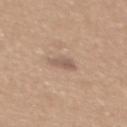Assessment: This lesion was catalogued during total-body skin photography and was not selected for biopsy. Background: On the mid back. A female patient in their 40s. Automated tile analysis of the lesion measured a footprint of about 3.5 mm² and an outline eccentricity of about 0.75 (0 = round, 1 = elongated). It also reported a mean CIELAB color near L≈57 a*≈17 b*≈26, roughly 9 lightness units darker than nearby skin, and a normalized border contrast of about 6.5. It also reported lesion-presence confidence of about 100/100. The lesion's longest dimension is about 2.5 mm. Imaged with white-light lighting. A roughly 15 mm field-of-view crop from a total-body skin photograph.